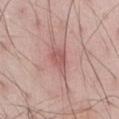Q: Was a biopsy performed?
A: imaged on a skin check; not biopsied
Q: Lesion size?
A: ~3.5 mm (longest diameter)
Q: How was the tile lit?
A: white-light
Q: Where on the body is the lesion?
A: the abdomen
Q: Who is the patient?
A: male, roughly 55 years of age
Q: How was this image acquired?
A: ~15 mm crop, total-body skin-cancer survey